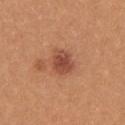Background:
The subject is a female aged 23 to 27. From the right upper arm. Cropped from a whole-body photographic skin survey; the tile spans about 15 mm.